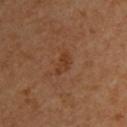follow-up = catalogued during a skin exam; not biopsied